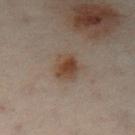No biopsy was performed on this lesion — it was imaged during a full skin examination and was not determined to be concerning.
A male subject, roughly 50 years of age.
Located on the left lower leg.
A region of skin cropped from a whole-body photographic capture, roughly 15 mm wide.